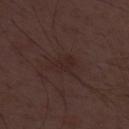biopsy_status: not biopsied; imaged during a skin examination
lighting: white-light
lesion_size:
  long_diameter_mm_approx: 3.5
automated_metrics:
  cielab_L: 24
  cielab_a: 16
  cielab_b: 17
  vs_skin_darker_L: 4.0
  vs_skin_contrast_norm: 4.5
  border_irregularity_0_10: 2.5
  color_variation_0_10: 2.0
  nevus_likeness_0_100: 0
image:
  source: total-body photography crop
  field_of_view_mm: 15
patient:
  sex: male
  age_approx: 50